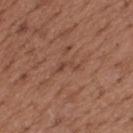workup=imaged on a skin check; not biopsied | subject=male, roughly 65 years of age | diameter=~3 mm (longest diameter) | imaging modality=15 mm crop, total-body photography | site=the upper back.